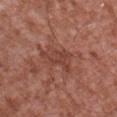<lesion>
<biopsy_status>not biopsied; imaged during a skin examination</biopsy_status>
<image>
  <source>total-body photography crop</source>
  <field_of_view_mm>15</field_of_view_mm>
</image>
<site>chest</site>
<patient>
  <sex>male</sex>
  <age_approx>45</age_approx>
</patient>
</lesion>